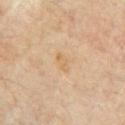notes: no biopsy performed (imaged during a skin exam)
image: 15 mm crop, total-body photography
site: the front of the torso
subject: male, in their mid- to late 60s